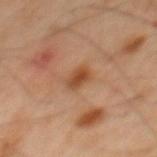| key | value |
|---|---|
| workup | total-body-photography surveillance lesion; no biopsy |
| body site | the mid back |
| imaging modality | ~15 mm crop, total-body skin-cancer survey |
| subject | male, aged approximately 45 |